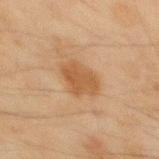Captured during whole-body skin photography for melanoma surveillance; the lesion was not biopsied.
The lesion's longest dimension is about 4 mm.
Cropped from a total-body skin-imaging series; the visible field is about 15 mm.
A male patient aged approximately 45.
The lesion is on the back.